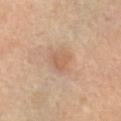Recorded during total-body skin imaging; not selected for excision or biopsy.
About 3 mm across.
Cropped from a total-body skin-imaging series; the visible field is about 15 mm.
An algorithmic analysis of the crop reported a footprint of about 6 mm², an outline eccentricity of about 0.25 (0 = round, 1 = elongated), and a symmetry-axis asymmetry near 0.25. It also reported border irregularity of about 2 on a 0–10 scale, a color-variation rating of about 1.5/10, and peripheral color asymmetry of about 0.5.
A male patient, about 65 years old.
Imaged with cross-polarized lighting.
From the chest.